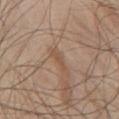Notes:
* follow-up: total-body-photography surveillance lesion; no biopsy
* body site: the chest
* image: ~15 mm tile from a whole-body skin photo
* illumination: white-light illumination
* patient: male, in their 50s
* lesion diameter: ≈3 mm